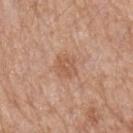Clinical impression:
The lesion was photographed on a routine skin check and not biopsied; there is no pathology result.
Clinical summary:
A 15 mm close-up tile from a total-body photography series done for melanoma screening. Located on the arm. An algorithmic analysis of the crop reported a footprint of about 6 mm², an eccentricity of roughly 0.5, and a symmetry-axis asymmetry near 0.2. The analysis additionally found an average lesion color of about L≈57 a*≈21 b*≈32 (CIELAB) and a normalized border contrast of about 5.5. The analysis additionally found border irregularity of about 2 on a 0–10 scale and internal color variation of about 2.5 on a 0–10 scale. A male subject, aged approximately 50.